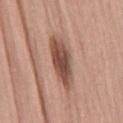Part of a total-body skin-imaging series; this lesion was reviewed on a skin check and was not flagged for biopsy. Captured under white-light illumination. A 15 mm close-up tile from a total-body photography series done for melanoma screening. An algorithmic analysis of the crop reported a lesion area of about 14 mm² and an eccentricity of roughly 0.95. The software also gave an automated nevus-likeness rating near 85 out of 100. The lesion's longest dimension is about 7 mm. The lesion is located on the lower back. The subject is a male aged 43–47.